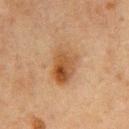Q: Was a biopsy performed?
A: total-body-photography surveillance lesion; no biopsy
Q: Patient demographics?
A: male, roughly 75 years of age
Q: What is the anatomic site?
A: the chest
Q: What is the imaging modality?
A: ~15 mm tile from a whole-body skin photo